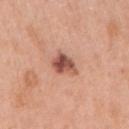About 3.5 mm across.
The lesion-visualizer software estimated a footprint of about 6 mm², an eccentricity of roughly 0.8, and a shape-asymmetry score of about 0.3 (0 = symmetric). It also reported a mean CIELAB color near L≈53 a*≈26 b*≈29, roughly 15 lightness units darker than nearby skin, and a normalized border contrast of about 10.
A lesion tile, about 15 mm wide, cut from a 3D total-body photograph.
The patient is a male in their 60s.
The lesion is located on the right upper arm.